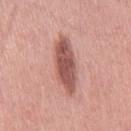Q: Was this lesion biopsied?
A: imaged on a skin check; not biopsied
Q: Illumination type?
A: white-light
Q: Where on the body is the lesion?
A: the right thigh
Q: Lesion size?
A: ~7 mm (longest diameter)
Q: How was this image acquired?
A: ~15 mm crop, total-body skin-cancer survey
Q: What are the patient's age and sex?
A: female, aged 38 to 42